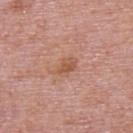Recorded during total-body skin imaging; not selected for excision or biopsy.
A male patient about 75 years old.
On the upper back.
A lesion tile, about 15 mm wide, cut from a 3D total-body photograph.